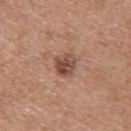Captured during whole-body skin photography for melanoma surveillance; the lesion was not biopsied.
A lesion tile, about 15 mm wide, cut from a 3D total-body photograph.
A male subject, aged around 30.
Automated image analysis of the tile measured an area of roughly 6.5 mm² and a shape-asymmetry score of about 0.2 (0 = symmetric). The analysis additionally found a border-irregularity index near 2/10, internal color variation of about 6 on a 0–10 scale, and radial color variation of about 2.
The lesion is located on the upper back.